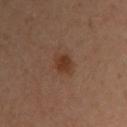follow-up = catalogued during a skin exam; not biopsied
patient = male, aged 38 to 42
imaging modality = 15 mm crop, total-body photography
anatomic site = the right upper arm